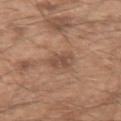The total-body-photography lesion software estimated a lesion area of about 4.5 mm², an eccentricity of roughly 0.55, and a shape-asymmetry score of about 0.35 (0 = symmetric). The analysis additionally found an average lesion color of about L≈49 a*≈19 b*≈28 (CIELAB) and a normalized border contrast of about 6.5. It also reported an automated nevus-likeness rating near 5 out of 100 and a detector confidence of about 100 out of 100 that the crop contains a lesion.
A male patient aged 48 to 52.
From the left upper arm.
A roughly 15 mm field-of-view crop from a total-body skin photograph.
Imaged with white-light lighting.
About 2.5 mm across.